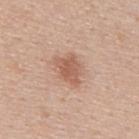workup: catalogued during a skin exam; not biopsied
image-analysis metrics: a border-irregularity index near 3.5/10 and radial color variation of about 0.5
anatomic site: the upper back
patient: male, roughly 40 years of age
imaging modality: ~15 mm tile from a whole-body skin photo
lighting: white-light illumination
size: about 3.5 mm